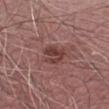  biopsy_status: not biopsied; imaged during a skin examination
  patient:
    sex: male
    age_approx: 70
  lighting: white-light
  site: right forearm
  image:
    source: total-body photography crop
    field_of_view_mm: 15